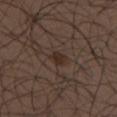Imaged during a routine full-body skin examination; the lesion was not biopsied and no histopathology is available. The lesion is located on the upper back. A 15 mm close-up tile from a total-body photography series done for melanoma screening. Captured under white-light illumination. The subject is a male aged approximately 30.